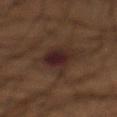Recorded during total-body skin imaging; not selected for excision or biopsy. The lesion is on the abdomen. The total-body-photography lesion software estimated a footprint of about 10 mm² and a symmetry-axis asymmetry near 0.3. And it measured a lesion–skin lightness drop of about 8 and a normalized border contrast of about 11. It also reported a classifier nevus-likeness of about 65/100 and lesion-presence confidence of about 95/100. Approximately 6 mm at its widest. Cropped from a whole-body photographic skin survey; the tile spans about 15 mm. The tile uses cross-polarized illumination. A male subject, aged 53–57.